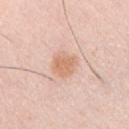This lesion was catalogued during total-body skin photography and was not selected for biopsy. A 15 mm close-up extracted from a 3D total-body photography capture. The patient is a male aged around 35. On the chest. This is a white-light tile. Longest diameter approximately 3 mm. The total-body-photography lesion software estimated a classifier nevus-likeness of about 70/100 and a detector confidence of about 100 out of 100 that the crop contains a lesion.